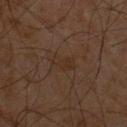Captured during whole-body skin photography for melanoma surveillance; the lesion was not biopsied. The patient is a male aged around 60. The lesion's longest dimension is about 3 mm. Cropped from a whole-body photographic skin survey; the tile spans about 15 mm. On the chest. An algorithmic analysis of the crop reported a mean CIELAB color near L≈26 a*≈14 b*≈23, about 4 CIELAB-L* units darker than the surrounding skin, and a lesion-to-skin contrast of about 5 (normalized; higher = more distinct). It also reported a border-irregularity index near 3/10 and a peripheral color-asymmetry measure near 0.5. The software also gave a classifier nevus-likeness of about 0/100 and a lesion-detection confidence of about 100/100.